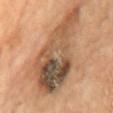patient = male, about 70 years old | acquisition = ~15 mm crop, total-body skin-cancer survey | location = the mid back.